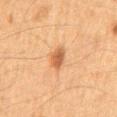patient=male, roughly 65 years of age; image source=15 mm crop, total-body photography; site=the mid back.